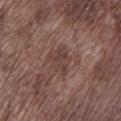Assessment: The lesion was tiled from a total-body skin photograph and was not biopsied. Clinical summary: Cropped from a whole-body photographic skin survey; the tile spans about 15 mm. On the left lower leg. The subject is a male about 75 years old. The lesion's longest dimension is about 3.5 mm. Automated tile analysis of the lesion measured a nevus-likeness score of about 0/100 and lesion-presence confidence of about 95/100. The tile uses white-light illumination.